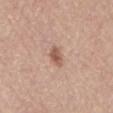Assessment:
Part of a total-body skin-imaging series; this lesion was reviewed on a skin check and was not flagged for biopsy.
Clinical summary:
About 2.5 mm across. Automated image analysis of the tile measured a shape eccentricity near 0.8. The software also gave a nevus-likeness score of about 80/100 and a detector confidence of about 100 out of 100 that the crop contains a lesion. A 15 mm close-up extracted from a 3D total-body photography capture. The subject is a male roughly 80 years of age. This is a white-light tile. From the mid back.